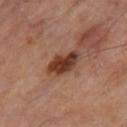biopsy status: catalogued during a skin exam; not biopsied
anatomic site: the right thigh
imaging modality: ~15 mm crop, total-body skin-cancer survey